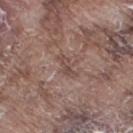Q: Was this lesion biopsied?
A: imaged on a skin check; not biopsied
Q: Lesion size?
A: about 2.5 mm
Q: How was this image acquired?
A: ~15 mm crop, total-body skin-cancer survey
Q: What lighting was used for the tile?
A: white-light illumination
Q: Lesion location?
A: the right thigh
Q: Who is the patient?
A: male, aged around 75